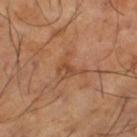• biopsy status · imaged on a skin check; not biopsied
• TBP lesion metrics · a footprint of about 5.5 mm²; an average lesion color of about L≈48 a*≈22 b*≈33 (CIELAB), a lesion–skin lightness drop of about 7, and a normalized lesion–skin contrast near 5.5; a border-irregularity index near 7/10, internal color variation of about 5 on a 0–10 scale, and radial color variation of about 2; a nevus-likeness score of about 0/100 and lesion-presence confidence of about 100/100
• subject · male, in their mid-60s
• site · the left thigh
• image · ~15 mm tile from a whole-body skin photo